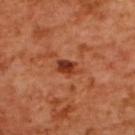Impression:
Imaged during a routine full-body skin examination; the lesion was not biopsied and no histopathology is available.
Clinical summary:
About 3 mm across. The lesion is located on the upper back. A female subject about 55 years old. This is a cross-polarized tile. A region of skin cropped from a whole-body photographic capture, roughly 15 mm wide.